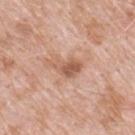Acquisition and patient details: A male subject, about 50 years old. A close-up tile cropped from a whole-body skin photograph, about 15 mm across. On the upper back. The lesion-visualizer software estimated a shape eccentricity near 0.8. The software also gave roughly 11 lightness units darker than nearby skin and a lesion-to-skin contrast of about 7.5 (normalized; higher = more distinct).